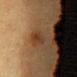The lesion was photographed on a routine skin check and not biopsied; there is no pathology result. A female patient roughly 55 years of age. The total-body-photography lesion software estimated an area of roughly 4.5 mm², a shape eccentricity near 0.95, and a shape-asymmetry score of about 0.3 (0 = symmetric). The analysis additionally found peripheral color asymmetry of about 0.5. And it measured a nevus-likeness score of about 35/100 and lesion-presence confidence of about 100/100. On the front of the torso. A close-up tile cropped from a whole-body skin photograph, about 15 mm across.